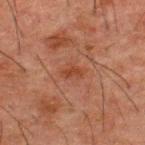Impression: The lesion was tiled from a total-body skin photograph and was not biopsied.